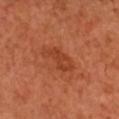biopsy status: imaged on a skin check; not biopsied | site: the head or neck | patient: male, aged around 50 | automated metrics: an area of roughly 7.5 mm² and a shape eccentricity near 0.75; a lesion color around L≈42 a*≈30 b*≈37 in CIELAB, about 7 CIELAB-L* units darker than the surrounding skin, and a lesion-to-skin contrast of about 5.5 (normalized; higher = more distinct); a nevus-likeness score of about 30/100 and a detector confidence of about 100 out of 100 that the crop contains a lesion | diameter: about 4 mm | image source: 15 mm crop, total-body photography | illumination: cross-polarized illumination.